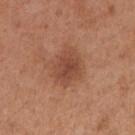Captured during whole-body skin photography for melanoma surveillance; the lesion was not biopsied.